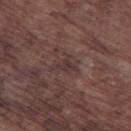This lesion was catalogued during total-body skin photography and was not selected for biopsy.
A male subject, aged 73–77.
The total-body-photography lesion software estimated an area of roughly 3.5 mm², an outline eccentricity of about 0.75 (0 = round, 1 = elongated), and a symmetry-axis asymmetry near 0.55. It also reported a lesion color around L≈34 a*≈17 b*≈16 in CIELAB and a lesion–skin lightness drop of about 6.
The lesion's longest dimension is about 3 mm.
This is a white-light tile.
A lesion tile, about 15 mm wide, cut from a 3D total-body photograph.
Located on the leg.